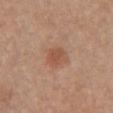Part of a total-body skin-imaging series; this lesion was reviewed on a skin check and was not flagged for biopsy.
The lesion is on the chest.
A close-up tile cropped from a whole-body skin photograph, about 15 mm across.
Approximately 3 mm at its widest.
This is a white-light tile.
A female patient, approximately 55 years of age.